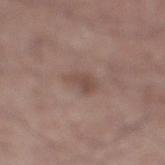Image and clinical context: A lesion tile, about 15 mm wide, cut from a 3D total-body photograph. Longest diameter approximately 3.5 mm. A male subject roughly 55 years of age. Automated image analysis of the tile measured a lesion area of about 5.5 mm², an eccentricity of roughly 0.8, and a symmetry-axis asymmetry near 0.3. The software also gave an average lesion color of about L≈48 a*≈17 b*≈23 (CIELAB), about 8 CIELAB-L* units darker than the surrounding skin, and a lesion-to-skin contrast of about 6 (normalized; higher = more distinct). It also reported border irregularity of about 3 on a 0–10 scale, a within-lesion color-variation index near 2/10, and radial color variation of about 0.5. The software also gave lesion-presence confidence of about 100/100. The lesion is located on the right lower leg.